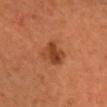Assessment: No biopsy was performed on this lesion — it was imaged during a full skin examination and was not determined to be concerning. Acquisition and patient details: A 15 mm crop from a total-body photograph taken for skin-cancer surveillance. About 3.5 mm across. Captured under cross-polarized illumination. From the head or neck. The subject is a female aged 43 to 47. The total-body-photography lesion software estimated an area of roughly 7.5 mm², an outline eccentricity of about 0.65 (0 = round, 1 = elongated), and two-axis asymmetry of about 0.3. And it measured a lesion color around L≈43 a*≈27 b*≈37 in CIELAB, about 11 CIELAB-L* units darker than the surrounding skin, and a lesion-to-skin contrast of about 8 (normalized; higher = more distinct). The software also gave a classifier nevus-likeness of about 85/100 and a detector confidence of about 100 out of 100 that the crop contains a lesion.